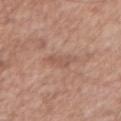workup — imaged on a skin check; not biopsied | imaging modality — ~15 mm crop, total-body skin-cancer survey | subject — male, aged 53 to 57 | lighting — white-light illumination | body site — the right upper arm | lesion diameter — about 3 mm | TBP lesion metrics — an area of roughly 3.5 mm².